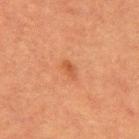Q: What is the anatomic site?
A: the back
Q: What kind of image is this?
A: ~15 mm crop, total-body skin-cancer survey
Q: What are the patient's age and sex?
A: male, about 50 years old